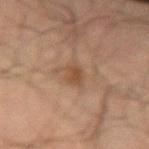biopsy status: total-body-photography surveillance lesion; no biopsy
acquisition: ~15 mm tile from a whole-body skin photo
lighting: cross-polarized
lesion size: about 3 mm
subject: male, roughly 70 years of age
body site: the left forearm
automated lesion analysis: a border-irregularity rating of about 2.5/10, a within-lesion color-variation index near 2/10, and radial color variation of about 0.5; an automated nevus-likeness rating near 50 out of 100